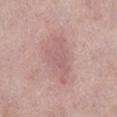Clinical impression: The lesion was photographed on a routine skin check and not biopsied; there is no pathology result. Background: A lesion tile, about 15 mm wide, cut from a 3D total-body photograph. From the leg. The subject is a female aged 38–42.